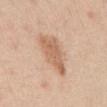Captured during whole-body skin photography for melanoma surveillance; the lesion was not biopsied. Automated image analysis of the tile measured a within-lesion color-variation index near 2.5/10. The software also gave an automated nevus-likeness rating near 50 out of 100 and a lesion-detection confidence of about 100/100. From the abdomen. About 6 mm across. A male subject, about 45 years old. A 15 mm close-up tile from a total-body photography series done for melanoma screening. Captured under white-light illumination.